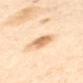No biopsy was performed on this lesion — it was imaged during a full skin examination and was not determined to be concerning. Imaged with cross-polarized lighting. A male patient, roughly 65 years of age. The total-body-photography lesion software estimated a lesion area of about 7 mm², an eccentricity of roughly 0.65, and two-axis asymmetry of about 0.25. It also reported a mean CIELAB color near L≈72 a*≈20 b*≈38 and a normalized border contrast of about 8. Longest diameter approximately 3.5 mm. A region of skin cropped from a whole-body photographic capture, roughly 15 mm wide. From the mid back.